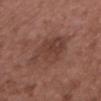Clinical impression:
No biopsy was performed on this lesion — it was imaged during a full skin examination and was not determined to be concerning.
Image and clinical context:
A male patient, aged around 50. This is a white-light tile. The lesion is located on the chest. The lesion-visualizer software estimated a footprint of about 15 mm², a shape eccentricity near 0.85, and two-axis asymmetry of about 0.25. And it measured a mean CIELAB color near L≈40 a*≈21 b*≈25 and about 8 CIELAB-L* units darker than the surrounding skin. And it measured a border-irregularity index near 4.5/10 and internal color variation of about 3.5 on a 0–10 scale. And it measured a classifier nevus-likeness of about 5/100. A 15 mm crop from a total-body photograph taken for skin-cancer surveillance.